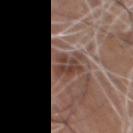| field | value |
|---|---|
| notes | catalogued during a skin exam; not biopsied |
| image source | ~15 mm crop, total-body skin-cancer survey |
| body site | the front of the torso |
| diameter | ≈4 mm |
| patient | male, aged 68 to 72 |